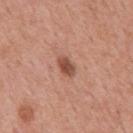follow-up: no biopsy performed (imaged during a skin exam)
illumination: white-light illumination
location: the mid back
subject: male, aged approximately 55
image: ~15 mm tile from a whole-body skin photo
TBP lesion metrics: an area of roughly 4 mm², an eccentricity of roughly 0.8, and a shape-asymmetry score of about 0.15 (0 = symmetric); a lesion color around L≈50 a*≈24 b*≈29 in CIELAB, about 12 CIELAB-L* units darker than the surrounding skin, and a normalized border contrast of about 8.5; a border-irregularity index near 1.5/10 and radial color variation of about 1; a classifier nevus-likeness of about 85/100 and a detector confidence of about 100 out of 100 that the crop contains a lesion
lesion diameter: about 3 mm